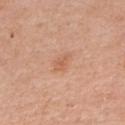Case summary:
– biopsy status: imaged on a skin check; not biopsied
– anatomic site: the right upper arm
– diameter: ≈3 mm
– image: ~15 mm tile from a whole-body skin photo
– automated metrics: an area of roughly 4 mm², an outline eccentricity of about 0.8 (0 = round, 1 = elongated), and two-axis asymmetry of about 0.35; an average lesion color of about L≈61 a*≈23 b*≈33 (CIELAB), about 7 CIELAB-L* units darker than the surrounding skin, and a normalized border contrast of about 5; border irregularity of about 3.5 on a 0–10 scale and peripheral color asymmetry of about 0.5; an automated nevus-likeness rating near 10 out of 100 and lesion-presence confidence of about 100/100
– tile lighting: white-light illumination
– patient: female, approximately 65 years of age